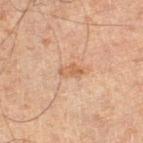Q: What kind of image is this?
A: total-body-photography crop, ~15 mm field of view
Q: What is the lesion's diameter?
A: ≈2.5 mm
Q: What are the patient's age and sex?
A: male, about 65 years old
Q: What did automated image analysis measure?
A: an area of roughly 3 mm², an outline eccentricity of about 0.85 (0 = round, 1 = elongated), and a symmetry-axis asymmetry near 0.3; an average lesion color of about L≈52 a*≈19 b*≈31 (CIELAB), roughly 8 lightness units darker than nearby skin, and a normalized border contrast of about 6
Q: What lighting was used for the tile?
A: cross-polarized illumination
Q: Lesion location?
A: the leg